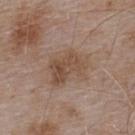This is a white-light tile. Longest diameter approximately 5 mm. From the upper back. This image is a 15 mm lesion crop taken from a total-body photograph. A male patient, approximately 55 years of age. The total-body-photography lesion software estimated a footprint of about 17 mm², a shape eccentricity near 0.65, and a symmetry-axis asymmetry near 0.2. It also reported a lesion color around L≈50 a*≈16 b*≈27 in CIELAB and a normalized lesion–skin contrast near 6.5. And it measured a classifier nevus-likeness of about 0/100 and a lesion-detection confidence of about 100/100.